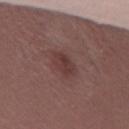<case>
<biopsy_status>not biopsied; imaged during a skin examination</biopsy_status>
</case>